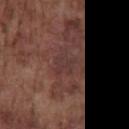workup: imaged on a skin check; not biopsied
illumination: white-light illumination
site: the chest
subject: male, in their mid-70s
imaging modality: total-body-photography crop, ~15 mm field of view